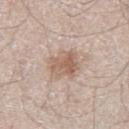follow-up: total-body-photography surveillance lesion; no biopsy
image-analysis metrics: a footprint of about 9 mm², an eccentricity of roughly 0.55, and a shape-asymmetry score of about 0.25 (0 = symmetric); a nevus-likeness score of about 95/100 and lesion-presence confidence of about 100/100
subject: male, aged 58 to 62
image source: ~15 mm crop, total-body skin-cancer survey
diameter: ≈4 mm
location: the right thigh
tile lighting: white-light illumination